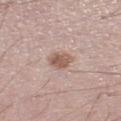patient: male, aged approximately 50; imaging modality: total-body-photography crop, ~15 mm field of view; location: the left lower leg; illumination: white-light; lesion size: about 3 mm.